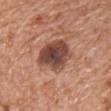Q: Is there a histopathology result?
A: total-body-photography surveillance lesion; no biopsy
Q: What did automated image analysis measure?
A: a lesion area of about 15 mm², a shape eccentricity near 0.8, and two-axis asymmetry of about 0.2; a border-irregularity index near 2.5/10, a within-lesion color-variation index near 6/10, and a peripheral color-asymmetry measure near 1.5; a nevus-likeness score of about 10/100 and a lesion-detection confidence of about 100/100
Q: What lighting was used for the tile?
A: white-light
Q: What are the patient's age and sex?
A: male, aged 63 to 67
Q: What kind of image is this?
A: 15 mm crop, total-body photography
Q: What is the anatomic site?
A: the chest